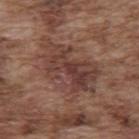notes: catalogued during a skin exam; not biopsied | image source: 15 mm crop, total-body photography | anatomic site: the upper back | patient: male, aged 73–77.